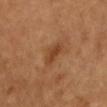Q: Was a biopsy performed?
A: imaged on a skin check; not biopsied
Q: What are the patient's age and sex?
A: male, about 55 years old
Q: What is the anatomic site?
A: the chest
Q: How was this image acquired?
A: total-body-photography crop, ~15 mm field of view